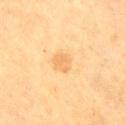Recorded during total-body skin imaging; not selected for excision or biopsy.
The patient is a female roughly 55 years of age.
A 15 mm crop from a total-body photograph taken for skin-cancer surveillance.
The total-body-photography lesion software estimated a lesion area of about 4 mm² and two-axis asymmetry of about 0.15. It also reported a within-lesion color-variation index near 1.5/10 and a peripheral color-asymmetry measure near 0.5.
This is a cross-polarized tile.
From the right thigh.